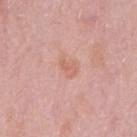Q: Is there a histopathology result?
A: total-body-photography surveillance lesion; no biopsy
Q: What is the imaging modality?
A: ~15 mm crop, total-body skin-cancer survey
Q: Illumination type?
A: white-light
Q: Patient demographics?
A: female, aged 48–52
Q: Lesion location?
A: the right upper arm
Q: Lesion size?
A: about 2.5 mm